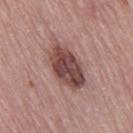No biopsy was performed on this lesion — it was imaged during a full skin examination and was not determined to be concerning.
A 15 mm crop from a total-body photograph taken for skin-cancer surveillance.
The subject is a female approximately 65 years of age.
Captured under white-light illumination.
The lesion's longest dimension is about 6.5 mm.
The lesion is on the right thigh.